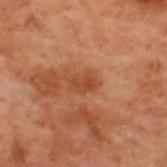Clinical summary:
The tile uses cross-polarized illumination. A male patient aged approximately 70. From the upper back. The recorded lesion diameter is about 2.5 mm. A lesion tile, about 15 mm wide, cut from a 3D total-body photograph. The total-body-photography lesion software estimated a mean CIELAB color near L≈34 a*≈21 b*≈29 and about 6 CIELAB-L* units darker than the surrounding skin. It also reported border irregularity of about 2 on a 0–10 scale and radial color variation of about 0.5.